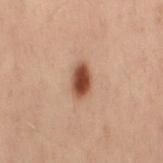The lesion was tiled from a total-body skin photograph and was not biopsied. On the lower back. A female subject in their mid-50s. A 15 mm close-up tile from a total-body photography series done for melanoma screening. An algorithmic analysis of the crop reported a footprint of about 6.5 mm², a shape eccentricity near 0.8, and a symmetry-axis asymmetry near 0.25. And it measured a mean CIELAB color near L≈41 a*≈19 b*≈27 and a lesion–skin lightness drop of about 14. The analysis additionally found a border-irregularity rating of about 2/10, internal color variation of about 4 on a 0–10 scale, and a peripheral color-asymmetry measure near 1. The software also gave a nevus-likeness score of about 100/100 and a lesion-detection confidence of about 100/100.